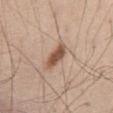Q: Was a biopsy performed?
A: no biopsy performed (imaged during a skin exam)
Q: What is the anatomic site?
A: the abdomen
Q: What lighting was used for the tile?
A: white-light
Q: Lesion size?
A: about 4 mm
Q: What are the patient's age and sex?
A: male, in their 40s
Q: What kind of image is this?
A: ~15 mm tile from a whole-body skin photo
Q: What did automated image analysis measure?
A: a lesion-detection confidence of about 100/100